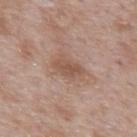Q: Was this lesion biopsied?
A: catalogued during a skin exam; not biopsied
Q: Where on the body is the lesion?
A: the mid back
Q: What is the imaging modality?
A: total-body-photography crop, ~15 mm field of view
Q: Lesion size?
A: about 3.5 mm
Q: Who is the patient?
A: male, aged around 55
Q: How was the tile lit?
A: white-light